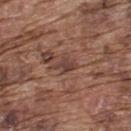Notes:
- imaging modality: 15 mm crop, total-body photography
- site: the upper back
- lesion diameter: about 3 mm
- TBP lesion metrics: a lesion area of about 3 mm² and a shape eccentricity near 0.8; a mean CIELAB color near L≈40 a*≈19 b*≈23 and a lesion–skin lightness drop of about 9; lesion-presence confidence of about 65/100
- lighting: white-light illumination
- patient: male, aged 73 to 77